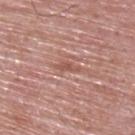biopsy status: no biopsy performed (imaged during a skin exam); patient: male, approximately 65 years of age; site: the upper back; lesion diameter: ≈3 mm; image source: ~15 mm tile from a whole-body skin photo.